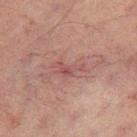biopsy_status: not biopsied; imaged during a skin examination
image:
  source: total-body photography crop
  field_of_view_mm: 15
automated_metrics:
  area_mm2_approx: 4.0
  eccentricity: 0.9
  shape_asymmetry: 0.6
  cielab_L: 37
  cielab_a: 20
  cielab_b: 18
  vs_skin_darker_L: 6.0
  vs_skin_contrast_norm: 5.5
  border_irregularity_0_10: 7.0
  color_variation_0_10: 1.0
  peripheral_color_asymmetry: 0.5
  lesion_detection_confidence_0_100: 100
patient:
  sex: male
  age_approx: 60
site: left leg
lighting: cross-polarized
lesion_size:
  long_diameter_mm_approx: 3.5